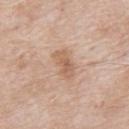Assessment:
No biopsy was performed on this lesion — it was imaged during a full skin examination and was not determined to be concerning.
Image and clinical context:
A male subject in their mid-60s. The lesion is on the upper back. A 15 mm crop from a total-body photograph taken for skin-cancer surveillance. About 3.5 mm across. This is a white-light tile.